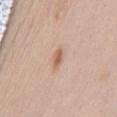A male patient, in their mid- to late 60s.
A close-up tile cropped from a whole-body skin photograph, about 15 mm across.
The lesion is located on the front of the torso.
This is a white-light tile.